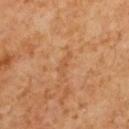No biopsy was performed on this lesion — it was imaged during a full skin examination and was not determined to be concerning. From the mid back. A roughly 15 mm field-of-view crop from a total-body skin photograph. A male patient, aged approximately 60. The total-body-photography lesion software estimated a lesion color around L≈50 a*≈21 b*≈37 in CIELAB, a lesion–skin lightness drop of about 5, and a lesion-to-skin contrast of about 4.5 (normalized; higher = more distinct). The software also gave internal color variation of about 0 on a 0–10 scale and a peripheral color-asymmetry measure near 0.